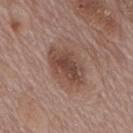Q: Illumination type?
A: white-light
Q: What kind of image is this?
A: 15 mm crop, total-body photography
Q: What is the anatomic site?
A: the mid back
Q: How large is the lesion?
A: about 5.5 mm
Q: Who is the patient?
A: male, aged 68–72